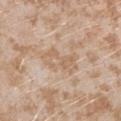{
  "biopsy_status": "not biopsied; imaged during a skin examination",
  "lighting": "white-light",
  "automated_metrics": {
    "area_mm2_approx": 9.5,
    "eccentricity": 0.8,
    "shape_asymmetry": 0.3,
    "border_irregularity_0_10": 4.5,
    "color_variation_0_10": 4.0,
    "peripheral_color_asymmetry": 1.5
  },
  "lesion_size": {
    "long_diameter_mm_approx": 4.5
  },
  "site": "left forearm",
  "patient": {
    "sex": "female",
    "age_approx": 25
  },
  "image": {
    "source": "total-body photography crop",
    "field_of_view_mm": 15
  }
}